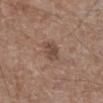From the right lower leg. A 15 mm close-up tile from a total-body photography series done for melanoma screening. The patient is a male aged approximately 65.